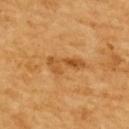Part of a total-body skin-imaging series; this lesion was reviewed on a skin check and was not flagged for biopsy. A female subject, in their mid- to late 50s. The tile uses cross-polarized illumination. A lesion tile, about 15 mm wide, cut from a 3D total-body photograph. The lesion-visualizer software estimated an area of roughly 7 mm², an outline eccentricity of about 0.9 (0 = round, 1 = elongated), and two-axis asymmetry of about 0.5. And it measured a nevus-likeness score of about 10/100. The lesion is on the back.